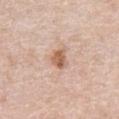workup — catalogued during a skin exam; not biopsied
diameter — about 2.5 mm
TBP lesion metrics — a lesion area of about 4.5 mm², a shape eccentricity near 0.55, and two-axis asymmetry of about 0.3; an average lesion color of about L≈61 a*≈21 b*≈31 (CIELAB), a lesion–skin lightness drop of about 12, and a lesion-to-skin contrast of about 8 (normalized; higher = more distinct); border irregularity of about 3 on a 0–10 scale, a within-lesion color-variation index near 3.5/10, and radial color variation of about 1.5; lesion-presence confidence of about 100/100
acquisition — total-body-photography crop, ~15 mm field of view
lighting — white-light
location — the chest
subject — female, aged approximately 60